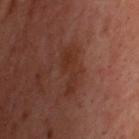{
  "biopsy_status": "not biopsied; imaged during a skin examination",
  "lighting": "cross-polarized",
  "automated_metrics": {
    "area_mm2_approx": 8.0,
    "eccentricity": 0.9,
    "shape_asymmetry": 0.45,
    "nevus_likeness_0_100": 0,
    "lesion_detection_confidence_0_100": 100
  },
  "lesion_size": {
    "long_diameter_mm_approx": 5.0
  },
  "patient": {
    "sex": "male",
    "age_approx": 40
  },
  "site": "upper back",
  "image": {
    "source": "total-body photography crop",
    "field_of_view_mm": 15
  }
}